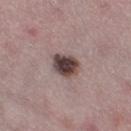follow-up: no biopsy performed (imaged during a skin exam)
lesion diameter: ~3.5 mm (longest diameter)
TBP lesion metrics: a lesion area of about 8 mm² and an outline eccentricity of about 0.35 (0 = round, 1 = elongated); a border-irregularity index near 1.5/10
location: the right thigh
lighting: white-light
imaging modality: 15 mm crop, total-body photography
subject: female, approximately 45 years of age